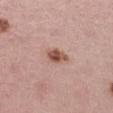The lesion was tiled from a total-body skin photograph and was not biopsied.
Longest diameter approximately 2.5 mm.
Located on the left thigh.
A female patient aged approximately 45.
A 15 mm crop from a total-body photograph taken for skin-cancer surveillance.